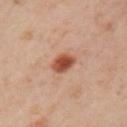Q: Is there a histopathology result?
A: no biopsy performed (imaged during a skin exam)
Q: What lighting was used for the tile?
A: cross-polarized
Q: Lesion location?
A: the left upper arm
Q: What is the imaging modality?
A: ~15 mm tile from a whole-body skin photo
Q: How large is the lesion?
A: ~3 mm (longest diameter)
Q: Patient demographics?
A: female, aged 38 to 42